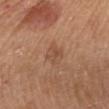Clinical impression:
Imaged during a routine full-body skin examination; the lesion was not biopsied and no histopathology is available.
Image and clinical context:
An algorithmic analysis of the crop reported roughly 6 lightness units darker than nearby skin and a lesion-to-skin contrast of about 5 (normalized; higher = more distinct). And it measured a border-irregularity index near 2/10, internal color variation of about 3 on a 0–10 scale, and a peripheral color-asymmetry measure near 1. The tile uses white-light illumination. The subject is a female aged 53–57. Located on the chest. Longest diameter approximately 2.5 mm. A 15 mm close-up tile from a total-body photography series done for melanoma screening.